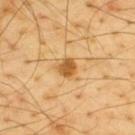No biopsy was performed on this lesion — it was imaged during a full skin examination and was not determined to be concerning. On the upper back. A lesion tile, about 15 mm wide, cut from a 3D total-body photograph. A male subject aged around 65. About 2.5 mm across.